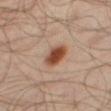workup: catalogued during a skin exam; not biopsied | subject: male, approximately 65 years of age | lesion size: ≈3.5 mm | tile lighting: cross-polarized illumination | acquisition: 15 mm crop, total-body photography | body site: the right thigh.